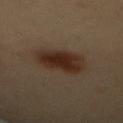{"biopsy_status": "not biopsied; imaged during a skin examination", "patient": {"sex": "female", "age_approx": 60}, "automated_metrics": {"cielab_L": 28, "cielab_a": 16, "cielab_b": 25, "vs_skin_darker_L": 10.0, "vs_skin_contrast_norm": 11.0, "border_irregularity_0_10": 2.0, "color_variation_0_10": 4.5, "nevus_likeness_0_100": 100, "lesion_detection_confidence_0_100": 100}, "lighting": "cross-polarized", "image": {"source": "total-body photography crop", "field_of_view_mm": 15}, "site": "mid back"}